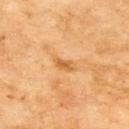{
  "biopsy_status": "not biopsied; imaged during a skin examination",
  "site": "upper back",
  "patient": {
    "sex": "male",
    "age_approx": 70
  },
  "lesion_size": {
    "long_diameter_mm_approx": 3.0
  },
  "lighting": "cross-polarized",
  "image": {
    "source": "total-body photography crop",
    "field_of_view_mm": 15
  },
  "automated_metrics": {
    "color_variation_0_10": 2.0,
    "peripheral_color_asymmetry": 0.5
  }
}